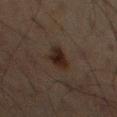workup = total-body-photography surveillance lesion; no biopsy
tile lighting = cross-polarized illumination
lesion diameter = ≈3.5 mm
image-analysis metrics = a lesion area of about 6 mm², an eccentricity of roughly 0.8, and two-axis asymmetry of about 0.3; a border-irregularity index near 2.5/10, a color-variation rating of about 4/10, and a peripheral color-asymmetry measure near 1; a classifier nevus-likeness of about 95/100 and a detector confidence of about 100 out of 100 that the crop contains a lesion
subject = male, in their mid- to late 60s
site = the right thigh
imaging modality = ~15 mm crop, total-body skin-cancer survey